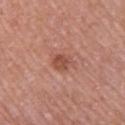Findings:
• workup: imaged on a skin check; not biopsied
• patient: female, in their mid- to late 60s
• anatomic site: the left upper arm
• acquisition: total-body-photography crop, ~15 mm field of view